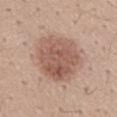Findings:
- workup: total-body-photography surveillance lesion; no biopsy
- automated metrics: a lesion area of about 28 mm², an eccentricity of roughly 0.55, and a symmetry-axis asymmetry near 0.15; a border-irregularity index near 2/10, internal color variation of about 5 on a 0–10 scale, and radial color variation of about 2; an automated nevus-likeness rating near 30 out of 100
- patient: male, aged approximately 40
- image: ~15 mm crop, total-body skin-cancer survey
- lesion size: ~6.5 mm (longest diameter)
- anatomic site: the lower back